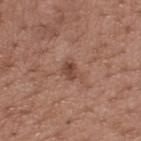Impression: Imaged during a routine full-body skin examination; the lesion was not biopsied and no histopathology is available. Acquisition and patient details: Located on the upper back. A roughly 15 mm field-of-view crop from a total-body skin photograph. Imaged with white-light lighting. The patient is a male in their mid-60s. Approximately 2.5 mm at its widest.